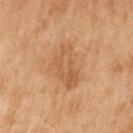Part of a total-body skin-imaging series; this lesion was reviewed on a skin check and was not flagged for biopsy.
A roughly 15 mm field-of-view crop from a total-body skin photograph.
The tile uses cross-polarized illumination.
From the left upper arm.
An algorithmic analysis of the crop reported a lesion area of about 12 mm², an outline eccentricity of about 0.9 (0 = round, 1 = elongated), and a shape-asymmetry score of about 0.35 (0 = symmetric).
A female patient approximately 70 years of age.
The lesion's longest dimension is about 6 mm.